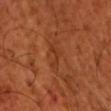Imaged during a routine full-body skin examination; the lesion was not biopsied and no histopathology is available.
A male patient about 50 years old.
Longest diameter approximately 3.5 mm.
Automated image analysis of the tile measured an area of roughly 3.5 mm², an outline eccentricity of about 0.9 (0 = round, 1 = elongated), and a symmetry-axis asymmetry near 0.65. The analysis additionally found radial color variation of about 0. It also reported an automated nevus-likeness rating near 0 out of 100 and lesion-presence confidence of about 60/100.
From the arm.
A 15 mm close-up tile from a total-body photography series done for melanoma screening.